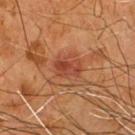The lesion was tiled from a total-body skin photograph and was not biopsied. A male patient, in their mid- to late 50s. The lesion is on the chest. A 15 mm close-up extracted from a 3D total-body photography capture.